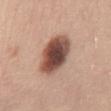Q: Who is the patient?
A: female, about 45 years old
Q: Illumination type?
A: white-light illumination
Q: What kind of image is this?
A: 15 mm crop, total-body photography
Q: What is the anatomic site?
A: the abdomen
Q: What is the lesion's diameter?
A: about 6 mm